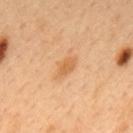Q: Was this lesion biopsied?
A: no biopsy performed (imaged during a skin exam)
Q: Where on the body is the lesion?
A: the mid back
Q: How was this image acquired?
A: ~15 mm tile from a whole-body skin photo
Q: Patient demographics?
A: male, aged 48 to 52
Q: What lighting was used for the tile?
A: cross-polarized
Q: How large is the lesion?
A: ~3 mm (longest diameter)
Q: Automated lesion metrics?
A: a lesion–skin lightness drop of about 8 and a normalized lesion–skin contrast near 6.5; lesion-presence confidence of about 100/100